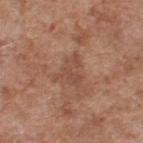  biopsy_status: not biopsied; imaged during a skin examination
  patient:
    sex: male
    age_approx: 60
  image:
    source: total-body photography crop
    field_of_view_mm: 15
  lighting: white-light
  automated_metrics:
    cielab_L: 48
    cielab_a: 21
    cielab_b: 29
    vs_skin_darker_L: 7.0
    vs_skin_contrast_norm: 5.0
    nevus_likeness_0_100: 0
    lesion_detection_confidence_0_100: 100
  site: upper back